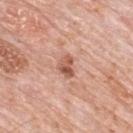<record>
<biopsy_status>not biopsied; imaged during a skin examination</biopsy_status>
</record>